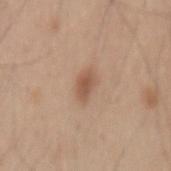biopsy status = imaged on a skin check; not biopsied | subject = male, in their mid-40s | site = the mid back | imaging modality = ~15 mm tile from a whole-body skin photo.